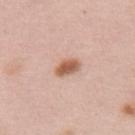| key | value |
|---|---|
| lesion diameter | about 3 mm |
| image-analysis metrics | a border-irregularity index near 2.5/10, internal color variation of about 2.5 on a 0–10 scale, and radial color variation of about 0.5; an automated nevus-likeness rating near 100 out of 100 and a detector confidence of about 100 out of 100 that the crop contains a lesion |
| patient | female, aged approximately 65 |
| lighting | white-light illumination |
| location | the upper back |
| imaging modality | ~15 mm crop, total-body skin-cancer survey |